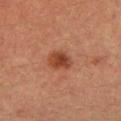Impression:
Recorded during total-body skin imaging; not selected for excision or biopsy.
Background:
Imaged with cross-polarized lighting. Automated tile analysis of the lesion measured an eccentricity of roughly 0.6. And it measured a nevus-likeness score of about 95/100 and lesion-presence confidence of about 100/100. The subject is a female approximately 55 years of age. The lesion is located on the left forearm. A 15 mm crop from a total-body photograph taken for skin-cancer surveillance.